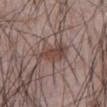{
  "biopsy_status": "not biopsied; imaged during a skin examination",
  "patient": {
    "sex": "male",
    "age_approx": 65
  },
  "image": {
    "source": "total-body photography crop",
    "field_of_view_mm": 15
  },
  "site": "abdomen"
}